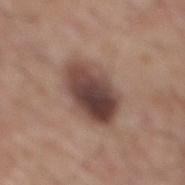This lesion was catalogued during total-body skin photography and was not selected for biopsy.
A lesion tile, about 15 mm wide, cut from a 3D total-body photograph.
Captured under white-light illumination.
A male patient aged approximately 60.
An algorithmic analysis of the crop reported an average lesion color of about L≈43 a*≈18 b*≈22 (CIELAB), roughly 15 lightness units darker than nearby skin, and a normalized lesion–skin contrast near 11.5. The analysis additionally found an automated nevus-likeness rating near 65 out of 100 and a lesion-detection confidence of about 100/100.
On the mid back.
The recorded lesion diameter is about 6.5 mm.